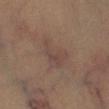Clinical impression: The lesion was photographed on a routine skin check and not biopsied; there is no pathology result. Context: A male subject, aged 43–47. Captured under cross-polarized illumination. An algorithmic analysis of the crop reported a lesion area of about 9 mm² and an eccentricity of roughly 0.8. And it measured an average lesion color of about L≈40 a*≈15 b*≈20 (CIELAB), about 5 CIELAB-L* units darker than the surrounding skin, and a lesion-to-skin contrast of about 4.5 (normalized; higher = more distinct). The software also gave border irregularity of about 5 on a 0–10 scale and radial color variation of about 1. Cropped from a total-body skin-imaging series; the visible field is about 15 mm. The recorded lesion diameter is about 4.5 mm. Located on the right lower leg.